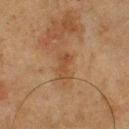| feature | finding |
|---|---|
| workup | catalogued during a skin exam; not biopsied |
| automated metrics | an average lesion color of about L≈37 a*≈18 b*≈30 (CIELAB), a lesion–skin lightness drop of about 6, and a lesion-to-skin contrast of about 6 (normalized; higher = more distinct); border irregularity of about 4 on a 0–10 scale and a color-variation rating of about 0.5/10 |
| subject | male, aged approximately 75 |
| size | about 3 mm |
| image source | ~15 mm crop, total-body skin-cancer survey |
| lighting | cross-polarized illumination |
| site | the front of the torso |